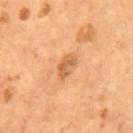<case>
  <biopsy_status>not biopsied; imaged during a skin examination</biopsy_status>
  <site>mid back</site>
  <patient>
    <sex>male</sex>
    <age_approx>55</age_approx>
  </patient>
  <image>
    <source>total-body photography crop</source>
    <field_of_view_mm>15</field_of_view_mm>
  </image>
</case>